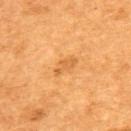Clinical impression: No biopsy was performed on this lesion — it was imaged during a full skin examination and was not determined to be concerning.